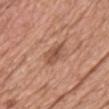Imaged during a routine full-body skin examination; the lesion was not biopsied and no histopathology is available.
The lesion is located on the chest.
A male subject, roughly 60 years of age.
Cropped from a whole-body photographic skin survey; the tile spans about 15 mm.
Imaged with white-light lighting.
Approximately 3.5 mm at its widest.